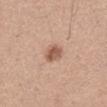Q: What kind of image is this?
A: ~15 mm tile from a whole-body skin photo
Q: What lighting was used for the tile?
A: white-light illumination
Q: How large is the lesion?
A: about 3 mm
Q: Lesion location?
A: the abdomen
Q: Who is the patient?
A: male, aged approximately 50